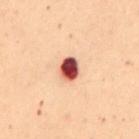Q: Was a biopsy performed?
A: no biopsy performed (imaged during a skin exam)
Q: How large is the lesion?
A: ≈3 mm
Q: What are the patient's age and sex?
A: female, aged around 50
Q: What lighting was used for the tile?
A: cross-polarized
Q: What is the imaging modality?
A: ~15 mm tile from a whole-body skin photo
Q: What did automated image analysis measure?
A: a lesion area of about 5.5 mm², a shape eccentricity near 0.65, and a shape-asymmetry score of about 0.3 (0 = symmetric); a normalized lesion–skin contrast near 16.5; border irregularity of about 2.5 on a 0–10 scale and peripheral color asymmetry of about 3; a classifier nevus-likeness of about 0/100
Q: Where on the body is the lesion?
A: the mid back